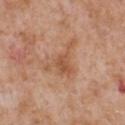Captured during whole-body skin photography for melanoma surveillance; the lesion was not biopsied. On the chest. Captured under white-light illumination. A roughly 15 mm field-of-view crop from a total-body skin photograph. About 5.5 mm across. A male patient aged 63 to 67.